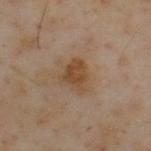follow-up: catalogued during a skin exam; not biopsied | subject: male, aged 53–57 | anatomic site: the upper back | lesion size: ~3.5 mm (longest diameter) | imaging modality: ~15 mm tile from a whole-body skin photo.